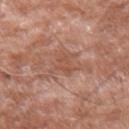Clinical impression:
Part of a total-body skin-imaging series; this lesion was reviewed on a skin check and was not flagged for biopsy.
Image and clinical context:
Cropped from a total-body skin-imaging series; the visible field is about 15 mm. Approximately 3 mm at its widest. A male subject aged 58–62. Located on the left forearm.